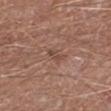The lesion was photographed on a routine skin check and not biopsied; there is no pathology result. The recorded lesion diameter is about 2.5 mm. A male patient in their 80s. The lesion is located on the left lower leg. The tile uses white-light illumination. A 15 mm close-up extracted from a 3D total-body photography capture.